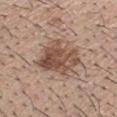Clinical impression:
Captured during whole-body skin photography for melanoma surveillance; the lesion was not biopsied.
Acquisition and patient details:
Automated tile analysis of the lesion measured about 13 CIELAB-L* units darker than the surrounding skin and a normalized lesion–skin contrast near 9. And it measured a border-irregularity rating of about 3.5/10, internal color variation of about 6.5 on a 0–10 scale, and radial color variation of about 2.5. The software also gave a classifier nevus-likeness of about 60/100 and lesion-presence confidence of about 100/100. A male subject, aged 28–32. Imaged with white-light lighting. The lesion's longest dimension is about 6.5 mm. Cropped from a total-body skin-imaging series; the visible field is about 15 mm. The lesion is located on the head or neck.